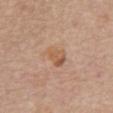This lesion was catalogued during total-body skin photography and was not selected for biopsy.